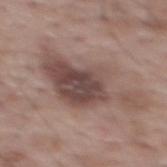This lesion was catalogued during total-body skin photography and was not selected for biopsy. A 15 mm close-up extracted from a 3D total-body photography capture. An algorithmic analysis of the crop reported a lesion color around L≈47 a*≈17 b*≈21 in CIELAB. And it measured border irregularity of about 7.5 on a 0–10 scale, a within-lesion color-variation index near 5/10, and a peripheral color-asymmetry measure near 1.5. The lesion's longest dimension is about 10 mm. This is a white-light tile. A male subject roughly 70 years of age. The lesion is located on the upper back.